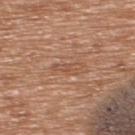  biopsy_status: not biopsied; imaged during a skin examination